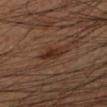The lesion was tiled from a total-body skin photograph and was not biopsied. On the left lower leg. A male subject, aged 43–47. A close-up tile cropped from a whole-body skin photograph, about 15 mm across. The lesion's longest dimension is about 4 mm.